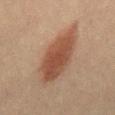Captured during whole-body skin photography for melanoma surveillance; the lesion was not biopsied. The tile uses cross-polarized illumination. The recorded lesion diameter is about 8 mm. Located on the mid back. A 15 mm close-up extracted from a 3D total-body photography capture. A male subject aged 58–62.